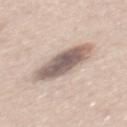Part of a total-body skin-imaging series; this lesion was reviewed on a skin check and was not flagged for biopsy. This is a white-light tile. The recorded lesion diameter is about 6.5 mm. A male subject, aged 43–47. Located on the mid back. A close-up tile cropped from a whole-body skin photograph, about 15 mm across.